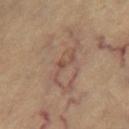site: right thigh
patient:
  sex: female
  age_approx: 65
image:
  source: total-body photography crop
  field_of_view_mm: 15
lesion_size:
  long_diameter_mm_approx: 5.0
lighting: cross-polarized